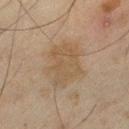{"biopsy_status": "not biopsied; imaged during a skin examination"}